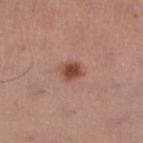Located on the left lower leg. A female subject, aged 53 to 57. The tile uses white-light illumination. A 15 mm close-up tile from a total-body photography series done for melanoma screening. Approximately 2.5 mm at its widest. The lesion-visualizer software estimated a lesion area of about 4.5 mm², an eccentricity of roughly 0.5, and two-axis asymmetry of about 0.2. It also reported a lesion–skin lightness drop of about 12 and a normalized border contrast of about 9.